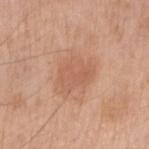The lesion was photographed on a routine skin check and not biopsied; there is no pathology result. A male subject roughly 60 years of age. Imaged with white-light lighting. Located on the right upper arm. About 4.5 mm across. A 15 mm close-up extracted from a 3D total-body photography capture.